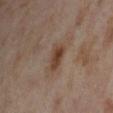{
  "automated_metrics": {
    "cielab_L": 42,
    "cielab_a": 18,
    "cielab_b": 27,
    "vs_skin_darker_L": 10.0,
    "vs_skin_contrast_norm": 9.0,
    "border_irregularity_0_10": 3.0,
    "color_variation_0_10": 3.0,
    "peripheral_color_asymmetry": 1.0
  },
  "patient": {
    "sex": "female",
    "age_approx": 55
  },
  "image": {
    "source": "total-body photography crop",
    "field_of_view_mm": 15
  },
  "lighting": "cross-polarized",
  "site": "right lower leg",
  "lesion_size": {
    "long_diameter_mm_approx": 3.5
  }
}